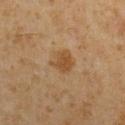Impression: The lesion was tiled from a total-body skin photograph and was not biopsied. Acquisition and patient details: Automated image analysis of the tile measured roughly 7 lightness units darker than nearby skin and a lesion-to-skin contrast of about 7 (normalized; higher = more distinct). The software also gave lesion-presence confidence of about 100/100. This is a cross-polarized tile. The subject is a male about 60 years old. About 2.5 mm across. A 15 mm crop from a total-body photograph taken for skin-cancer surveillance. Located on the chest.